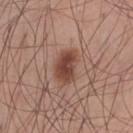* notes: total-body-photography surveillance lesion; no biopsy
* patient: male, aged around 30
* anatomic site: the chest
* image-analysis metrics: a lesion area of about 8 mm², an outline eccentricity of about 0.8 (0 = round, 1 = elongated), and two-axis asymmetry of about 0.25; a mean CIELAB color near L≈44 a*≈22 b*≈27 and a normalized border contrast of about 9.5; a nevus-likeness score of about 100/100 and a detector confidence of about 100 out of 100 that the crop contains a lesion
* size: about 4 mm
* image source: 15 mm crop, total-body photography
* tile lighting: white-light